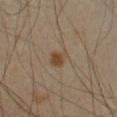No biopsy was performed on this lesion — it was imaged during a full skin examination and was not determined to be concerning.